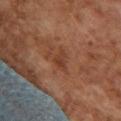A region of skin cropped from a whole-body photographic capture, roughly 15 mm wide. A female subject aged 58 to 62. Captured under cross-polarized illumination. The lesion is located on the upper back. Longest diameter approximately 3 mm.